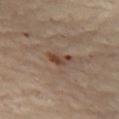Captured during whole-body skin photography for melanoma surveillance; the lesion was not biopsied. From the left thigh. Longest diameter approximately 3 mm. A 15 mm close-up extracted from a 3D total-body photography capture. A female patient, in their 60s. Captured under cross-polarized illumination.